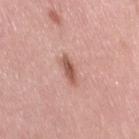Part of a total-body skin-imaging series; this lesion was reviewed on a skin check and was not flagged for biopsy. A female patient, about 50 years old. Approximately 3.5 mm at its widest. A lesion tile, about 15 mm wide, cut from a 3D total-body photograph. Imaged with white-light lighting. Located on the right thigh.